Located on the upper back. This is a white-light tile. A male subject in their 60s. This image is a 15 mm lesion crop taken from a total-body photograph:
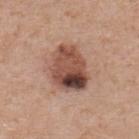Histopathology of the biopsied lesion showed a melanoma in situ arising in association with a nevus.